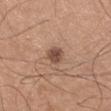biopsy_status: not biopsied; imaged during a skin examination
image:
  source: total-body photography crop
  field_of_view_mm: 15
lesion_size:
  long_diameter_mm_approx: 2.5
site: left upper arm
lighting: white-light
patient:
  sex: male
  age_approx: 25